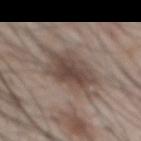Assessment:
Recorded during total-body skin imaging; not selected for excision or biopsy.